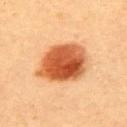Q: Is there a histopathology result?
A: catalogued during a skin exam; not biopsied
Q: Lesion location?
A: the upper back
Q: Who is the patient?
A: female, aged around 60
Q: Lesion size?
A: about 6.5 mm
Q: What lighting was used for the tile?
A: cross-polarized
Q: How was this image acquired?
A: ~15 mm crop, total-body skin-cancer survey